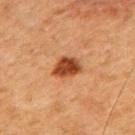notes — catalogued during a skin exam; not biopsied | imaging modality — 15 mm crop, total-body photography | illumination — cross-polarized | body site — the back | patient — male, aged 48 to 52.